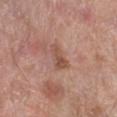<case>
  <image>
    <source>total-body photography crop</source>
    <field_of_view_mm>15</field_of_view_mm>
  </image>
  <lesion_size>
    <long_diameter_mm_approx>3.0</long_diameter_mm_approx>
  </lesion_size>
  <patient>
    <sex>male</sex>
    <age_approx>80</age_approx>
  </patient>
  <site>right lower leg</site>
</case>